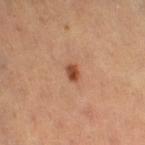biopsy status: total-body-photography surveillance lesion; no biopsy | TBP lesion metrics: an area of roughly 3 mm², an outline eccentricity of about 0.75 (0 = round, 1 = elongated), and a symmetry-axis asymmetry near 0.25; a normalized lesion–skin contrast near 9.5; a color-variation rating of about 3.5/10; a detector confidence of about 100 out of 100 that the crop contains a lesion | patient: male, roughly 65 years of age | image source: total-body-photography crop, ~15 mm field of view | anatomic site: the right thigh | diameter: ~2 mm (longest diameter).